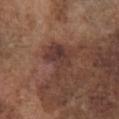<record>
  <biopsy_status>not biopsied; imaged during a skin examination</biopsy_status>
  <automated_metrics>
    <cielab_L>37</cielab_L>
    <cielab_a>19</cielab_a>
    <cielab_b>22</cielab_b>
    <vs_skin_darker_L>7.0</vs_skin_darker_L>
    <border_irregularity_0_10>6.0</border_irregularity_0_10>
    <color_variation_0_10>5.5</color_variation_0_10>
  </automated_metrics>
  <lesion_size>
    <long_diameter_mm_approx>4.5</long_diameter_mm_approx>
  </lesion_size>
  <site>chest</site>
  <image>
    <source>total-body photography crop</source>
    <field_of_view_mm>15</field_of_view_mm>
  </image>
  <patient>
    <sex>male</sex>
    <age_approx>75</age_approx>
  </patient>
</record>